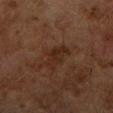Imaged during a routine full-body skin examination; the lesion was not biopsied and no histopathology is available.
A female subject in their 60s.
A close-up tile cropped from a whole-body skin photograph, about 15 mm across.
About 4 mm across.
Captured under cross-polarized illumination.
From the right upper arm.